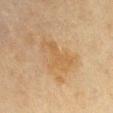– notes — total-body-photography surveillance lesion; no biopsy
– image — total-body-photography crop, ~15 mm field of view
– tile lighting — cross-polarized
– location — the left upper arm
– lesion size — about 5 mm
– subject — male, aged around 70